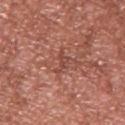follow-up: catalogued during a skin exam; not biopsied
patient: male, about 45 years old
lesion size: about 3 mm
body site: the chest
tile lighting: white-light illumination
image source: ~15 mm crop, total-body skin-cancer survey